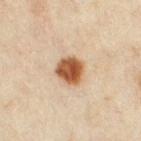| feature | finding |
|---|---|
| workup | catalogued during a skin exam; not biopsied |
| image source | total-body-photography crop, ~15 mm field of view |
| location | the right thigh |
| subject | female, in their mid-30s |
| diameter | ~3.5 mm (longest diameter) |
| tile lighting | cross-polarized illumination |